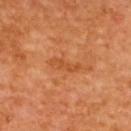Impression:
The lesion was tiled from a total-body skin photograph and was not biopsied.
Image and clinical context:
The tile uses cross-polarized illumination. Cropped from a total-body skin-imaging series; the visible field is about 15 mm. The lesion's longest dimension is about 3.5 mm. The total-body-photography lesion software estimated a footprint of about 3 mm² and two-axis asymmetry of about 0.45. The analysis additionally found a border-irregularity rating of about 7/10, a color-variation rating of about 0/10, and a peripheral color-asymmetry measure near 0. The software also gave an automated nevus-likeness rating near 0 out of 100 and lesion-presence confidence of about 100/100. Located on the upper back. A female subject about 65 years old.